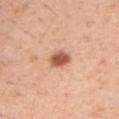follow-up: imaged on a skin check; not biopsied | lesion size: ~3 mm (longest diameter) | body site: the left upper arm | subject: male, roughly 40 years of age | imaging modality: total-body-photography crop, ~15 mm field of view.